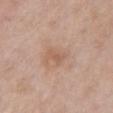Captured during whole-body skin photography for melanoma surveillance; the lesion was not biopsied.
The lesion is on the chest.
The tile uses white-light illumination.
The patient is a female aged around 50.
The lesion's longest dimension is about 2.5 mm.
A roughly 15 mm field-of-view crop from a total-body skin photograph.